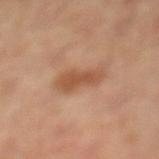<tbp_lesion>
  <biopsy_status>not biopsied; imaged during a skin examination</biopsy_status>
  <site>left lower leg</site>
  <patient>
    <sex>female</sex>
    <age_approx>60</age_approx>
  </patient>
  <lighting>cross-polarized</lighting>
  <image>
    <source>total-body photography crop</source>
    <field_of_view_mm>15</field_of_view_mm>
  </image>
  <lesion_size>
    <long_diameter_mm_approx>4.5</long_diameter_mm_approx>
  </lesion_size>
  <automated_metrics>
    <border_irregularity_0_10>3.5</border_irregularity_0_10>
    <color_variation_0_10>2.5</color_variation_0_10>
    <peripheral_color_asymmetry>1.0</peripheral_color_asymmetry>
    <nevus_likeness_0_100>45</nevus_likeness_0_100>
  </automated_metrics>
</tbp_lesion>